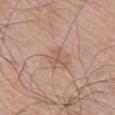Q: Lesion size?
A: about 2.5 mm
Q: Patient demographics?
A: male, aged 68–72
Q: What is the anatomic site?
A: the left arm
Q: What is the imaging modality?
A: 15 mm crop, total-body photography